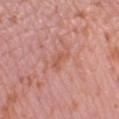Q: Was this lesion biopsied?
A: no biopsy performed (imaged during a skin exam)
Q: Where on the body is the lesion?
A: the leg
Q: How was this image acquired?
A: ~15 mm tile from a whole-body skin photo
Q: Who is the patient?
A: female, roughly 40 years of age